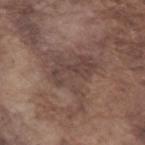Impression:
This lesion was catalogued during total-body skin photography and was not selected for biopsy.
Background:
Measured at roughly 6.5 mm in maximum diameter. A lesion tile, about 15 mm wide, cut from a 3D total-body photograph. A male patient approximately 75 years of age. The lesion is located on the left forearm. The tile uses white-light illumination.